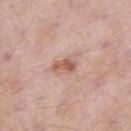notes=no biopsy performed (imaged during a skin exam) | automated metrics=an average lesion color of about L≈57 a*≈23 b*≈27 (CIELAB), roughly 12 lightness units darker than nearby skin, and a normalized lesion–skin contrast near 8; a within-lesion color-variation index near 1.5/10 and radial color variation of about 0; an automated nevus-likeness rating near 45 out of 100 and a detector confidence of about 100 out of 100 that the crop contains a lesion | subject=male, approximately 55 years of age | acquisition=15 mm crop, total-body photography | tile lighting=white-light illumination | location=the right thigh.